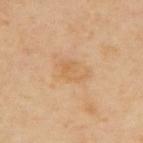The lesion was tiled from a total-body skin photograph and was not biopsied.
Captured under cross-polarized illumination.
About 3.5 mm across.
Cropped from a total-body skin-imaging series; the visible field is about 15 mm.
The subject is a male in their mid- to late 60s.
On the upper back.